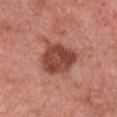Captured during whole-body skin photography for melanoma surveillance; the lesion was not biopsied. A female subject, about 60 years old. Automated image analysis of the tile measured a footprint of about 16 mm², an eccentricity of roughly 0.45, and two-axis asymmetry of about 0.25. It also reported a border-irregularity rating of about 2.5/10, a within-lesion color-variation index near 4.5/10, and radial color variation of about 1.5. The lesion's longest dimension is about 5 mm. Cropped from a total-body skin-imaging series; the visible field is about 15 mm. The tile uses white-light illumination. The lesion is located on the chest.